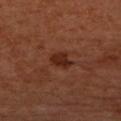follow-up=catalogued during a skin exam; not biopsied
patient=female, approximately 65 years of age
site=the left forearm
lesion size=≈2.5 mm
tile lighting=cross-polarized illumination
image=~15 mm tile from a whole-body skin photo